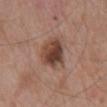Q: Was a biopsy performed?
A: catalogued during a skin exam; not biopsied
Q: What kind of image is this?
A: ~15 mm crop, total-body skin-cancer survey
Q: How was the tile lit?
A: white-light illumination
Q: Who is the patient?
A: male, roughly 80 years of age
Q: What is the lesion's diameter?
A: about 5 mm
Q: What is the anatomic site?
A: the mid back
Q: What did automated image analysis measure?
A: an area of roughly 12 mm² and two-axis asymmetry of about 0.2; about 13 CIELAB-L* units darker than the surrounding skin and a normalized lesion–skin contrast near 10; border irregularity of about 2.5 on a 0–10 scale and radial color variation of about 2.5; a nevus-likeness score of about 85/100 and a lesion-detection confidence of about 100/100